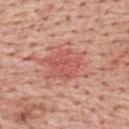No biopsy was performed on this lesion — it was imaged during a full skin examination and was not determined to be concerning. The patient is a male aged around 60. The tile uses white-light illumination. The lesion is on the upper back. Approximately 6.5 mm at its widest. The lesion-visualizer software estimated roughly 9 lightness units darker than nearby skin and a normalized lesion–skin contrast near 6. The analysis additionally found a nevus-likeness score of about 55/100 and a lesion-detection confidence of about 100/100. A close-up tile cropped from a whole-body skin photograph, about 15 mm across.